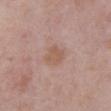| key | value |
|---|---|
| follow-up | no biopsy performed (imaged during a skin exam) |
| location | the front of the torso |
| lighting | white-light illumination |
| patient | male, aged around 55 |
| acquisition | 15 mm crop, total-body photography |